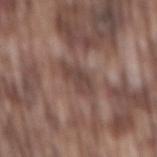– biopsy status — total-body-photography surveillance lesion; no biopsy
– location — the mid back
– TBP lesion metrics — a border-irregularity rating of about 2.5/10 and a within-lesion color-variation index near 2.5/10
– patient — male, in their mid-70s
– diameter — about 4 mm
– image — 15 mm crop, total-body photography